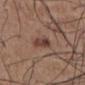Clinical impression: Captured during whole-body skin photography for melanoma surveillance; the lesion was not biopsied. Image and clinical context: This is a white-light tile. A roughly 15 mm field-of-view crop from a total-body skin photograph. The lesion is located on the abdomen. The recorded lesion diameter is about 3.5 mm. A male subject, approximately 55 years of age.